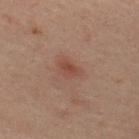Captured during whole-body skin photography for melanoma surveillance; the lesion was not biopsied. A 15 mm close-up extracted from a 3D total-body photography capture. Approximately 3 mm at its widest. The tile uses cross-polarized illumination. On the upper back. A male subject about 50 years old.